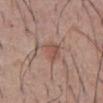Part of a total-body skin-imaging series; this lesion was reviewed on a skin check and was not flagged for biopsy.
A male patient aged 53–57.
Located on the abdomen.
Automated image analysis of the tile measured a mean CIELAB color near L≈53 a*≈19 b*≈25. The software also gave a detector confidence of about 100 out of 100 that the crop contains a lesion.
Imaged with white-light lighting.
A roughly 15 mm field-of-view crop from a total-body skin photograph.